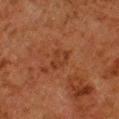Case summary:
• follow-up · total-body-photography surveillance lesion; no biopsy
• subject · male, about 80 years old
• anatomic site · the leg
• acquisition · ~15 mm crop, total-body skin-cancer survey